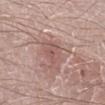subject = male, aged approximately 40 | lesion size = ≈3 mm | imaging modality = ~15 mm tile from a whole-body skin photo | lighting = white-light | anatomic site = the leg.